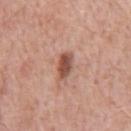<lesion>
  <image>
    <source>total-body photography crop</source>
    <field_of_view_mm>15</field_of_view_mm>
  </image>
  <patient>
    <sex>male</sex>
    <age_approx>60</age_approx>
  </patient>
  <site>chest</site>
  <lesion_size>
    <long_diameter_mm_approx>3.5</long_diameter_mm_approx>
  </lesion_size>
  <lighting>white-light</lighting>
</lesion>